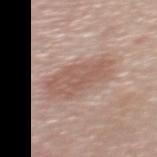workup=imaged on a skin check; not biopsied | anatomic site=the upper back | image source=total-body-photography crop, ~15 mm field of view | lesion diameter=≈6 mm | subject=female, roughly 50 years of age.